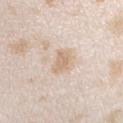Clinical impression: Recorded during total-body skin imaging; not selected for excision or biopsy. Clinical summary: Automated image analysis of the tile measured an area of roughly 4.5 mm², a shape eccentricity near 0.8, and two-axis asymmetry of about 0.3. And it measured an average lesion color of about L≈69 a*≈14 b*≈30 (CIELAB), roughly 9 lightness units darker than nearby skin, and a lesion-to-skin contrast of about 6.5 (normalized; higher = more distinct). It also reported a within-lesion color-variation index near 1.5/10 and a peripheral color-asymmetry measure near 0.5. It also reported a detector confidence of about 95 out of 100 that the crop contains a lesion. The recorded lesion diameter is about 3 mm. A region of skin cropped from a whole-body photographic capture, roughly 15 mm wide. The lesion is on the right forearm. The patient is a female in their mid-20s. Captured under white-light illumination.